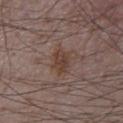The lesion was photographed on a routine skin check and not biopsied; there is no pathology result. A roughly 15 mm field-of-view crop from a total-body skin photograph. The lesion-visualizer software estimated an area of roughly 5 mm², an outline eccentricity of about 0.8 (0 = round, 1 = elongated), and two-axis asymmetry of about 0.25. The software also gave an average lesion color of about L≈40 a*≈17 b*≈23 (CIELAB), about 8 CIELAB-L* units darker than the surrounding skin, and a normalized lesion–skin contrast near 7.5. The analysis additionally found border irregularity of about 2.5 on a 0–10 scale, internal color variation of about 3 on a 0–10 scale, and radial color variation of about 1. The software also gave an automated nevus-likeness rating near 25 out of 100. The lesion is on the chest. The recorded lesion diameter is about 3 mm. Captured under white-light illumination. A male patient, in their mid- to late 70s.